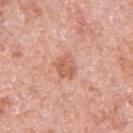Assessment:
Recorded during total-body skin imaging; not selected for excision or biopsy.
Image and clinical context:
On the left upper arm. The subject is a male in their 80s. The tile uses white-light illumination. A 15 mm crop from a total-body photograph taken for skin-cancer surveillance.